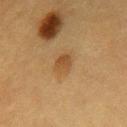Impression: No biopsy was performed on this lesion — it was imaged during a full skin examination and was not determined to be concerning. Context: The patient is a female in their mid- to late 50s. Imaged with cross-polarized lighting. The lesion-visualizer software estimated border irregularity of about 2 on a 0–10 scale, a color-variation rating of about 2.5/10, and radial color variation of about 1. The analysis additionally found a nevus-likeness score of about 85/100 and a detector confidence of about 100 out of 100 that the crop contains a lesion. The lesion is located on the chest. A 15 mm close-up tile from a total-body photography series done for melanoma screening.